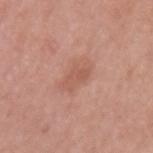notes: catalogued during a skin exam; not biopsied | acquisition: 15 mm crop, total-body photography | lighting: white-light | lesion size: ~4 mm (longest diameter) | automated metrics: border irregularity of about 3 on a 0–10 scale and internal color variation of about 2.5 on a 0–10 scale; a detector confidence of about 100 out of 100 that the crop contains a lesion | site: the left upper arm | subject: female, aged 48–52.